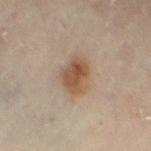follow-up — no biopsy performed (imaged during a skin exam).